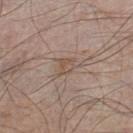<lesion>
  <lesion_size>
    <long_diameter_mm_approx>3.5</long_diameter_mm_approx>
  </lesion_size>
  <image>
    <source>total-body photography crop</source>
    <field_of_view_mm>15</field_of_view_mm>
  </image>
  <lighting>white-light</lighting>
  <patient>
    <sex>male</sex>
    <age_approx>65</age_approx>
  </patient>
  <site>left lower leg</site>
</lesion>